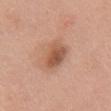The lesion was tiled from a total-body skin photograph and was not biopsied.
A female patient in their 50s.
Located on the chest.
A roughly 15 mm field-of-view crop from a total-body skin photograph.